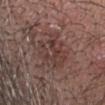No biopsy was performed on this lesion — it was imaged during a full skin examination and was not determined to be concerning. Cropped from a total-body skin-imaging series; the visible field is about 15 mm. Approximately 6.5 mm at its widest. A male subject, roughly 25 years of age. The lesion is on the head or neck.